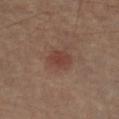No biopsy was performed on this lesion — it was imaged during a full skin examination and was not determined to be concerning. The lesion is located on the left forearm. A 15 mm close-up extracted from a 3D total-body photography capture. Measured at roughly 3 mm in maximum diameter. The total-body-photography lesion software estimated an area of roughly 6 mm² and an outline eccentricity of about 0.55 (0 = round, 1 = elongated). It also reported a mean CIELAB color near L≈39 a*≈21 b*≈24 and roughly 7 lightness units darker than nearby skin. And it measured a classifier nevus-likeness of about 75/100 and a detector confidence of about 100 out of 100 that the crop contains a lesion. A male patient aged 63 to 67. This is a cross-polarized tile.